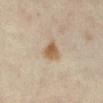<tbp_lesion>
<biopsy_status>not biopsied; imaged during a skin examination</biopsy_status>
<lighting>cross-polarized</lighting>
<lesion_size>
  <long_diameter_mm_approx>2.5</long_diameter_mm_approx>
</lesion_size>
<site>right lower leg</site>
<image>
  <source>total-body photography crop</source>
  <field_of_view_mm>15</field_of_view_mm>
</image>
<automated_metrics>
  <nevus_likeness_0_100>95</nevus_likeness_0_100>
</automated_metrics>
<patient>
  <sex>female</sex>
  <age_approx>45</age_approx>
</patient>
</tbp_lesion>